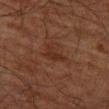biopsy status: total-body-photography surveillance lesion; no biopsy
patient: male, aged 78–82
tile lighting: cross-polarized
anatomic site: the left thigh
lesion size: ~3 mm (longest diameter)
acquisition: ~15 mm crop, total-body skin-cancer survey
image-analysis metrics: a normalized lesion–skin contrast near 6; a detector confidence of about 100 out of 100 that the crop contains a lesion